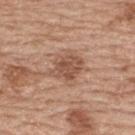  biopsy_status: not biopsied; imaged during a skin examination
  lighting: white-light
  site: back
  image:
    source: total-body photography crop
    field_of_view_mm: 15
  patient:
    sex: female
    age_approx: 60